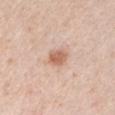No biopsy was performed on this lesion — it was imaged during a full skin examination and was not determined to be concerning. A 15 mm close-up tile from a total-body photography series done for melanoma screening. This is a white-light tile. The lesion is on the arm. A male patient, aged approximately 40. About 2.5 mm across.